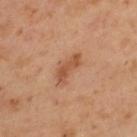{
  "biopsy_status": "not biopsied; imaged during a skin examination",
  "lighting": "cross-polarized",
  "patient": {
    "sex": "male",
    "age_approx": 55
  },
  "site": "upper back",
  "automated_metrics": {
    "eccentricity": 0.9,
    "shape_asymmetry": 0.35
  },
  "image": {
    "source": "total-body photography crop",
    "field_of_view_mm": 15
  },
  "lesion_size": {
    "long_diameter_mm_approx": 4.0
  }
}